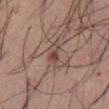Clinical impression: Part of a total-body skin-imaging series; this lesion was reviewed on a skin check and was not flagged for biopsy. Context: A roughly 15 mm field-of-view crop from a total-body skin photograph. An algorithmic analysis of the crop reported a lesion area of about 3 mm², an eccentricity of roughly 0.85, and a shape-asymmetry score of about 0.5 (0 = symmetric). And it measured a mean CIELAB color near L≈45 a*≈19 b*≈25, roughly 9 lightness units darker than nearby skin, and a lesion-to-skin contrast of about 7 (normalized; higher = more distinct). The software also gave a border-irregularity index near 5/10, a color-variation rating of about 1.5/10, and a peripheral color-asymmetry measure near 0.5. The lesion is on the abdomen. This is a white-light tile. A male patient, in their mid-50s.